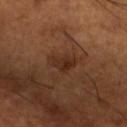| feature | finding |
|---|---|
| notes | no biopsy performed (imaged during a skin exam) |